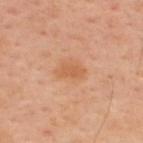| field | value |
|---|---|
| workup | catalogued during a skin exam; not biopsied |
| body site | the back |
| automated lesion analysis | two-axis asymmetry of about 0.35 |
| patient | male, roughly 45 years of age |
| image | ~15 mm crop, total-body skin-cancer survey |
| illumination | cross-polarized |
| diameter | about 3 mm |